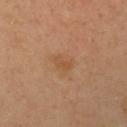Impression:
Recorded during total-body skin imaging; not selected for excision or biopsy.
Acquisition and patient details:
The lesion is located on the left upper arm. A female subject, about 40 years old. Captured under cross-polarized illumination. Automated image analysis of the tile measured a mean CIELAB color near L≈53 a*≈21 b*≈37, roughly 6 lightness units darker than nearby skin, and a normalized border contrast of about 5.5. And it measured a border-irregularity index near 3/10, a color-variation rating of about 2/10, and radial color variation of about 0.5. A region of skin cropped from a whole-body photographic capture, roughly 15 mm wide.